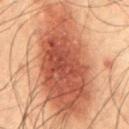biopsy status=imaged on a skin check; not biopsied | automated lesion analysis=a lesion area of about 70 mm², a shape eccentricity near 0.9, and two-axis asymmetry of about 0.2; a mean CIELAB color near L≈41 a*≈22 b*≈27 and a lesion–skin lightness drop of about 13; a classifier nevus-likeness of about 100/100 and lesion-presence confidence of about 100/100 | subject=male, aged approximately 50 | imaging modality=~15 mm tile from a whole-body skin photo | tile lighting=cross-polarized | lesion size=~14.5 mm (longest diameter) | body site=the abdomen.